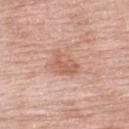| field | value |
|---|---|
| notes | total-body-photography surveillance lesion; no biopsy |
| TBP lesion metrics | a lesion area of about 7.5 mm² and a shape eccentricity near 0.7; border irregularity of about 3 on a 0–10 scale and a peripheral color-asymmetry measure near 1; a nevus-likeness score of about 5/100 and a detector confidence of about 100 out of 100 that the crop contains a lesion |
| subject | female, approximately 70 years of age |
| location | the upper back |
| lesion diameter | ~3.5 mm (longest diameter) |
| image source | ~15 mm tile from a whole-body skin photo |